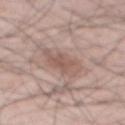follow-up: total-body-photography surveillance lesion; no biopsy | site: the back | patient: male, roughly 55 years of age | acquisition: ~15 mm tile from a whole-body skin photo | automated lesion analysis: a color-variation rating of about 3/10 and a peripheral color-asymmetry measure near 1 | lesion size: about 5 mm.